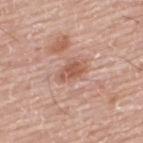{"biopsy_status": "not biopsied; imaged during a skin examination", "patient": {"sex": "male", "age_approx": 75}, "lighting": "white-light", "image": {"source": "total-body photography crop", "field_of_view_mm": 15}, "lesion_size": {"long_diameter_mm_approx": 3.0}, "site": "upper back"}